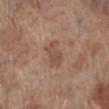<tbp_lesion>
  <biopsy_status>not biopsied; imaged during a skin examination</biopsy_status>
  <site>left lower leg</site>
  <patient>
    <sex>male</sex>
    <age_approx>70</age_approx>
  </patient>
  <image>
    <source>total-body photography crop</source>
    <field_of_view_mm>15</field_of_view_mm>
  </image>
  <lesion_size>
    <long_diameter_mm_approx>3.5</long_diameter_mm_approx>
  </lesion_size>
  <automated_metrics>
    <area_mm2_approx>7.0</area_mm2_approx>
  </automated_metrics>
  <lighting>white-light</lighting>
</tbp_lesion>